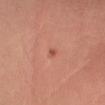The lesion was photographed on a routine skin check and not biopsied; there is no pathology result.
A roughly 15 mm field-of-view crop from a total-body skin photograph.
A male subject in their mid- to late 50s.
The lesion is on the left forearm.
Imaged with cross-polarized lighting.
The lesion's longest dimension is about 1 mm.